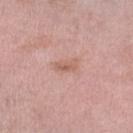Assessment:
Captured during whole-body skin photography for melanoma surveillance; the lesion was not biopsied.
Background:
The patient is a female aged 68 to 72. Cropped from a total-body skin-imaging series; the visible field is about 15 mm. From the left lower leg.